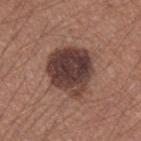  biopsy_status: not biopsied; imaged during a skin examination
  site: left forearm
  patient:
    sex: male
    age_approx: 35
  image:
    source: total-body photography crop
    field_of_view_mm: 15